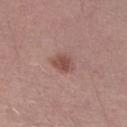The lesion was tiled from a total-body skin photograph and was not biopsied. A 15 mm crop from a total-body photograph taken for skin-cancer surveillance. A male subject aged approximately 30.